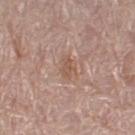This lesion was catalogued during total-body skin photography and was not selected for biopsy.
On the right thigh.
The tile uses white-light illumination.
About 2.5 mm across.
The total-body-photography lesion software estimated a footprint of about 4 mm², a shape eccentricity near 0.8, and a symmetry-axis asymmetry near 0.35. And it measured a border-irregularity rating of about 4/10 and a within-lesion color-variation index near 2/10. The analysis additionally found a detector confidence of about 100 out of 100 that the crop contains a lesion.
The subject is a female in their mid- to late 60s.
A 15 mm close-up tile from a total-body photography series done for melanoma screening.